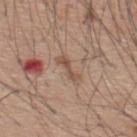  biopsy_status: not biopsied; imaged during a skin examination
  patient:
    sex: male
    age_approx: 60
  site: upper back
  automated_metrics:
    area_mm2_approx: 2.5
    shape_asymmetry: 0.4
  lighting: white-light
  image:
    source: total-body photography crop
    field_of_view_mm: 15
  lesion_size:
    long_diameter_mm_approx: 3.5